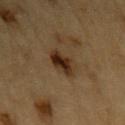follow-up = imaged on a skin check; not biopsied
lighting = cross-polarized illumination
size = ~3 mm (longest diameter)
subject = male, approximately 85 years of age or older
acquisition = ~15 mm tile from a whole-body skin photo
body site = the left upper arm